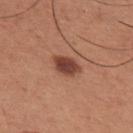Captured during whole-body skin photography for melanoma surveillance; the lesion was not biopsied. Imaged with white-light lighting. Automated tile analysis of the lesion measured a footprint of about 6 mm² and a shape eccentricity near 0.75. And it measured an average lesion color of about L≈43 a*≈24 b*≈27 (CIELAB) and a normalized border contrast of about 11. A male patient approximately 35 years of age. On the chest. A lesion tile, about 15 mm wide, cut from a 3D total-body photograph.